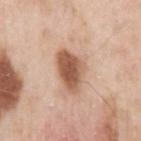Clinical impression: The lesion was tiled from a total-body skin photograph and was not biopsied. Image and clinical context: The subject is a male about 55 years old. The tile uses white-light illumination. A 15 mm close-up extracted from a 3D total-body photography capture. On the left upper arm. The total-body-photography lesion software estimated a lesion area of about 11 mm², an eccentricity of roughly 0.7, and a symmetry-axis asymmetry near 0.3. It also reported an automated nevus-likeness rating near 95 out of 100 and a detector confidence of about 100 out of 100 that the crop contains a lesion.